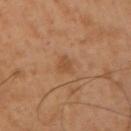biopsy_status: not biopsied; imaged during a skin examination
patient:
  sex: male
  age_approx: 50
image:
  source: total-body photography crop
  field_of_view_mm: 15
lighting: cross-polarized
site: right upper arm